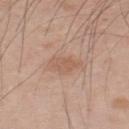patient — male, aged around 35
body site — the back
image — total-body-photography crop, ~15 mm field of view